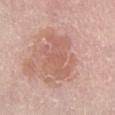| feature | finding |
|---|---|
| follow-up | total-body-photography surveillance lesion; no biopsy |
| imaging modality | total-body-photography crop, ~15 mm field of view |
| subject | female, in their mid- to late 60s |
| anatomic site | the right lower leg |